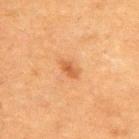Recorded during total-body skin imaging; not selected for excision or biopsy. The tile uses cross-polarized illumination. The subject is a male aged 48 to 52. The lesion's longest dimension is about 2.5 mm. A 15 mm crop from a total-body photograph taken for skin-cancer surveillance. Located on the upper back.